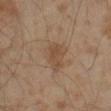Part of a total-body skin-imaging series; this lesion was reviewed on a skin check and was not flagged for biopsy. The lesion is on the right thigh. About 4 mm across. A male subject roughly 60 years of age. A 15 mm close-up extracted from a 3D total-body photography capture. This is a cross-polarized tile.